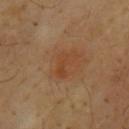Q: Is there a histopathology result?
A: catalogued during a skin exam; not biopsied
Q: Where on the body is the lesion?
A: the upper back
Q: What kind of image is this?
A: ~15 mm crop, total-body skin-cancer survey
Q: Lesion size?
A: ≈3.5 mm
Q: Who is the patient?
A: male, in their mid- to late 60s